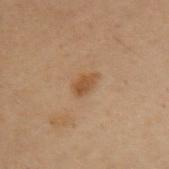| feature | finding |
|---|---|
| workup | imaged on a skin check; not biopsied |
| subject | female, in their 50s |
| diameter | ≈3 mm |
| automated lesion analysis | a footprint of about 4 mm², a shape eccentricity near 0.8, and a symmetry-axis asymmetry near 0.3 |
| lighting | cross-polarized |
| image | ~15 mm tile from a whole-body skin photo |
| site | the left upper arm |